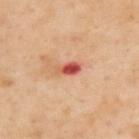Part of a total-body skin-imaging series; this lesion was reviewed on a skin check and was not flagged for biopsy. The total-body-photography lesion software estimated an eccentricity of roughly 0.85 and a shape-asymmetry score of about 0.2 (0 = symmetric). The software also gave an average lesion color of about L≈55 a*≈34 b*≈33 (CIELAB), roughly 15 lightness units darker than nearby skin, and a normalized lesion–skin contrast near 10. The analysis additionally found a border-irregularity rating of about 2/10, internal color variation of about 2.5 on a 0–10 scale, and peripheral color asymmetry of about 0.5. The subject is a male aged 53 to 57. The recorded lesion diameter is about 3 mm. The tile uses cross-polarized illumination. On the upper back. A region of skin cropped from a whole-body photographic capture, roughly 15 mm wide.